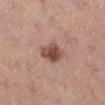{"biopsy_status": "not biopsied; imaged during a skin examination", "patient": {"sex": "female", "age_approx": 25}, "site": "right lower leg", "lesion_size": {"long_diameter_mm_approx": 4.0}, "automated_metrics": {"area_mm2_approx": 7.0, "eccentricity": 0.8, "shape_asymmetry": 0.25}, "image": {"source": "total-body photography crop", "field_of_view_mm": 15}}